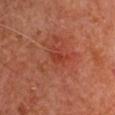The lesion was photographed on a routine skin check and not biopsied; there is no pathology result. From the left upper arm. Cropped from a total-body skin-imaging series; the visible field is about 15 mm. A male subject aged approximately 65. Automated image analysis of the tile measured an area of roughly 1 mm². The software also gave an average lesion color of about L≈34 a*≈30 b*≈31 (CIELAB) and a lesion-to-skin contrast of about 5.5 (normalized; higher = more distinct). The analysis additionally found a nevus-likeness score of about 0/100 and a detector confidence of about 100 out of 100 that the crop contains a lesion.